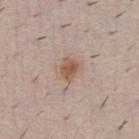Recorded during total-body skin imaging; not selected for excision or biopsy. Measured at roughly 2.5 mm in maximum diameter. Automated image analysis of the tile measured a lesion area of about 4.5 mm² and a symmetry-axis asymmetry near 0.3. And it measured a nevus-likeness score of about 90/100. A 15 mm close-up extracted from a 3D total-body photography capture. On the chest. A male subject about 40 years old.